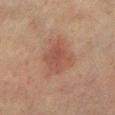Clinical impression: Imaged during a routine full-body skin examination; the lesion was not biopsied and no histopathology is available. Background: The lesion is on the leg. A male subject, in their mid-70s. Longest diameter approximately 4.5 mm. The tile uses cross-polarized illumination. A 15 mm crop from a total-body photograph taken for skin-cancer surveillance.